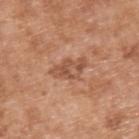Impression: This lesion was catalogued during total-body skin photography and was not selected for biopsy. Context: A male patient in their mid-60s. An algorithmic analysis of the crop reported an eccentricity of roughly 0.9 and two-axis asymmetry of about 0.3. The analysis additionally found a mean CIELAB color near L≈53 a*≈23 b*≈33 and a normalized border contrast of about 6.5. It also reported lesion-presence confidence of about 100/100. A lesion tile, about 15 mm wide, cut from a 3D total-body photograph. From the upper back. The tile uses white-light illumination. Approximately 4.5 mm at its widest.